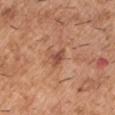The lesion was photographed on a routine skin check and not biopsied; there is no pathology result.
Measured at roughly 2.5 mm in maximum diameter.
A male subject, about 40 years old.
The tile uses white-light illumination.
On the arm.
Cropped from a total-body skin-imaging series; the visible field is about 15 mm.